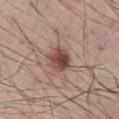Recorded during total-body skin imaging; not selected for excision or biopsy. A male subject, aged around 60. Cropped from a whole-body photographic skin survey; the tile spans about 15 mm. On the abdomen. Captured under white-light illumination.